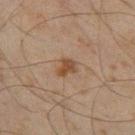Impression: No biopsy was performed on this lesion — it was imaged during a full skin examination and was not determined to be concerning. Image and clinical context: This image is a 15 mm lesion crop taken from a total-body photograph. On the left thigh. Approximately 2.5 mm at its widest. A male patient, aged approximately 45. Captured under cross-polarized illumination.